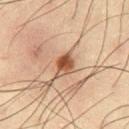Clinical impression: Recorded during total-body skin imaging; not selected for excision or biopsy. Clinical summary: A male patient, aged 33 to 37. The lesion is on the left thigh. A 15 mm crop from a total-body photograph taken for skin-cancer surveillance.